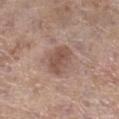This lesion was catalogued during total-body skin photography and was not selected for biopsy. A 15 mm crop from a total-body photograph taken for skin-cancer surveillance. The lesion-visualizer software estimated an outline eccentricity of about 0.6 (0 = round, 1 = elongated) and a symmetry-axis asymmetry near 0.15. And it measured a lesion color around L≈51 a*≈18 b*≈25 in CIELAB, about 10 CIELAB-L* units darker than the surrounding skin, and a lesion-to-skin contrast of about 7 (normalized; higher = more distinct). It also reported border irregularity of about 1.5 on a 0–10 scale, a color-variation rating of about 3/10, and peripheral color asymmetry of about 1. The recorded lesion diameter is about 3.5 mm. The patient is a female about 85 years old or older. Captured under white-light illumination. The lesion is located on the right lower leg.